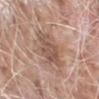Assessment:
The lesion was tiled from a total-body skin photograph and was not biopsied.
Clinical summary:
The recorded lesion diameter is about 5 mm. Cropped from a total-body skin-imaging series; the visible field is about 15 mm. A male patient in their mid- to late 70s. Captured under white-light illumination. An algorithmic analysis of the crop reported an average lesion color of about L≈53 a*≈19 b*≈26 (CIELAB), about 10 CIELAB-L* units darker than the surrounding skin, and a normalized lesion–skin contrast near 6.5. The software also gave a classifier nevus-likeness of about 0/100. The lesion is located on the left forearm.